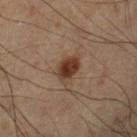No biopsy was performed on this lesion — it was imaged during a full skin examination and was not determined to be concerning.
A male subject aged 48 to 52.
From the right lower leg.
The recorded lesion diameter is about 3 mm.
Imaged with cross-polarized lighting.
A 15 mm close-up extracted from a 3D total-body photography capture.